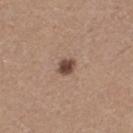Q: Was a biopsy performed?
A: total-body-photography surveillance lesion; no biopsy
Q: How was this image acquired?
A: 15 mm crop, total-body photography
Q: What lighting was used for the tile?
A: white-light illumination
Q: What is the anatomic site?
A: the left thigh
Q: How large is the lesion?
A: about 2 mm
Q: What are the patient's age and sex?
A: female, aged 43 to 47